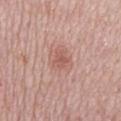follow-up: imaged on a skin check; not biopsied | imaging modality: ~15 mm crop, total-body skin-cancer survey | patient: male, aged 73–77 | body site: the mid back.